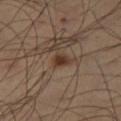No biopsy was performed on this lesion — it was imaged during a full skin examination and was not determined to be concerning.
Longest diameter approximately 2 mm.
From the right thigh.
A male patient, aged around 65.
A roughly 15 mm field-of-view crop from a total-body skin photograph.
Captured under cross-polarized illumination.
Automated image analysis of the tile measured a border-irregularity index near 2.5/10, internal color variation of about 2 on a 0–10 scale, and a peripheral color-asymmetry measure near 0.5. The analysis additionally found an automated nevus-likeness rating near 95 out of 100 and a lesion-detection confidence of about 100/100.